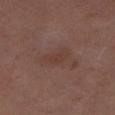follow-up: imaged on a skin check; not biopsied
acquisition: ~15 mm tile from a whole-body skin photo
diameter: about 3.5 mm
image-analysis metrics: a footprint of about 5.5 mm², a shape eccentricity near 0.85, and a shape-asymmetry score of about 0.35 (0 = symmetric); a lesion color around L≈36 a*≈18 b*≈22 in CIELAB, roughly 4 lightness units darker than nearby skin, and a normalized border contrast of about 5; a border-irregularity index near 3.5/10 and a color-variation rating of about 1.5/10; a classifier nevus-likeness of about 0/100 and lesion-presence confidence of about 100/100
illumination: cross-polarized
patient: female, about 50 years old
location: the leg